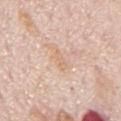Notes:
• notes · imaged on a skin check; not biopsied
• body site · the mid back
• TBP lesion metrics · a lesion color around L≈69 a*≈18 b*≈32 in CIELAB and a normalized lesion–skin contrast near 5; lesion-presence confidence of about 100/100
• subject · male, aged approximately 75
• tile lighting · white-light illumination
• imaging modality · total-body-photography crop, ~15 mm field of view
• size · ~2.5 mm (longest diameter)